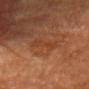This lesion was catalogued during total-body skin photography and was not selected for biopsy. On the head or neck. A male subject, roughly 85 years of age or older. A lesion tile, about 15 mm wide, cut from a 3D total-body photograph.